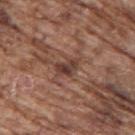No biopsy was performed on this lesion — it was imaged during a full skin examination and was not determined to be concerning.
On the back.
A close-up tile cropped from a whole-body skin photograph, about 15 mm across.
A male patient, approximately 75 years of age.
The tile uses white-light illumination.
Approximately 3 mm at its widest.
Automated image analysis of the tile measured a lesion area of about 4.5 mm² and an eccentricity of roughly 0.75. And it measured a border-irregularity rating of about 3/10, a color-variation rating of about 7.5/10, and a peripheral color-asymmetry measure near 2. The analysis additionally found a classifier nevus-likeness of about 0/100.